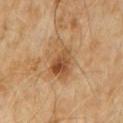Captured under cross-polarized illumination. Approximately 6 mm at its widest. Located on the abdomen. An algorithmic analysis of the crop reported a lesion area of about 12 mm², a shape eccentricity near 0.85, and a symmetry-axis asymmetry near 0.25. It also reported an average lesion color of about L≈50 a*≈19 b*≈36 (CIELAB), a lesion–skin lightness drop of about 10, and a lesion-to-skin contrast of about 8 (normalized; higher = more distinct). The analysis additionally found a border-irregularity index near 3.5/10 and radial color variation of about 2.5. The subject is a male roughly 60 years of age. A 15 mm close-up tile from a total-body photography series done for melanoma screening.